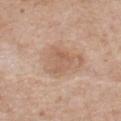<lesion>
  <biopsy_status>not biopsied; imaged during a skin examination</biopsy_status>
  <lesion_size>
    <long_diameter_mm_approx>3.5</long_diameter_mm_approx>
  </lesion_size>
  <lighting>white-light</lighting>
  <automated_metrics>
    <area_mm2_approx>8.5</area_mm2_approx>
    <eccentricity>0.5</eccentricity>
    <shape_asymmetry>0.3</shape_asymmetry>
    <peripheral_color_asymmetry>1.0</peripheral_color_asymmetry>
  </automated_metrics>
  <site>front of the torso</site>
  <image>
    <source>total-body photography crop</source>
    <field_of_view_mm>15</field_of_view_mm>
  </image>
  <patient>
    <sex>male</sex>
    <age_approx>80</age_approx>
  </patient>
</lesion>